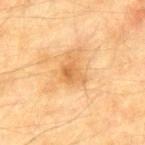Imaged during a routine full-body skin examination; the lesion was not biopsied and no histopathology is available.
The total-body-photography lesion software estimated a lesion color around L≈54 a*≈20 b*≈39 in CIELAB and roughly 8 lightness units darker than nearby skin. The analysis additionally found a within-lesion color-variation index near 3.5/10 and peripheral color asymmetry of about 1. And it measured an automated nevus-likeness rating near 0 out of 100.
A region of skin cropped from a whole-body photographic capture, roughly 15 mm wide.
A male subject, about 75 years old.
The lesion is on the mid back.